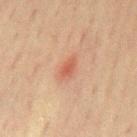Impression:
Part of a total-body skin-imaging series; this lesion was reviewed on a skin check and was not flagged for biopsy.
Clinical summary:
A male subject, aged 68 to 72. On the chest. This image is a 15 mm lesion crop taken from a total-body photograph.